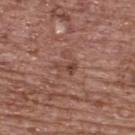Q: Was a biopsy performed?
A: catalogued during a skin exam; not biopsied
Q: Patient demographics?
A: female, aged around 65
Q: Lesion size?
A: about 2.5 mm
Q: How was this image acquired?
A: 15 mm crop, total-body photography
Q: What is the anatomic site?
A: the upper back
Q: What lighting was used for the tile?
A: white-light illumination